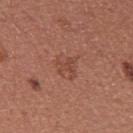The lesion was photographed on a routine skin check and not biopsied; there is no pathology result. A female patient approximately 25 years of age. The total-body-photography lesion software estimated about 6 CIELAB-L* units darker than the surrounding skin and a normalized border contrast of about 4.5. Longest diameter approximately 3 mm. Located on the upper back. Captured under white-light illumination. A 15 mm crop from a total-body photograph taken for skin-cancer surveillance.